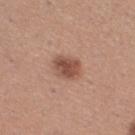Clinical impression: Recorded during total-body skin imaging; not selected for excision or biopsy. Context: This image is a 15 mm lesion crop taken from a total-body photograph. A female subject about 30 years old. Longest diameter approximately 3 mm. On the right thigh. This is a white-light tile.